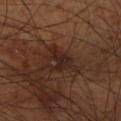Assessment:
The lesion was photographed on a routine skin check and not biopsied; there is no pathology result.
Context:
On the right lower leg. Automated tile analysis of the lesion measured a lesion area of about 5 mm², a shape eccentricity near 0.75, and a shape-asymmetry score of about 0.6 (0 = symmetric). The analysis additionally found a border-irregularity rating of about 7/10, a color-variation rating of about 2/10, and peripheral color asymmetry of about 0.5. It also reported a lesion-detection confidence of about 90/100. A male subject aged 68 to 72. A 15 mm close-up tile from a total-body photography series done for melanoma screening.